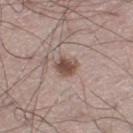{"biopsy_status": "not biopsied; imaged during a skin examination"}